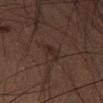On the right thigh.
A close-up tile cropped from a whole-body skin photograph, about 15 mm across.
A male patient aged around 55.
Captured under cross-polarized illumination.
The lesion's longest dimension is about 3 mm.